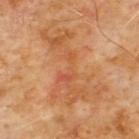Image and clinical context: The subject is a male aged 58 to 62. The lesion's longest dimension is about 10 mm. The lesion is on the front of the torso. Cropped from a total-body skin-imaging series; the visible field is about 15 mm. Imaged with cross-polarized lighting.